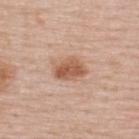Assessment: This lesion was catalogued during total-body skin photography and was not selected for biopsy. Acquisition and patient details: A female subject, roughly 50 years of age. About 4 mm across. From the upper back. This is a white-light tile. A region of skin cropped from a whole-body photographic capture, roughly 15 mm wide.